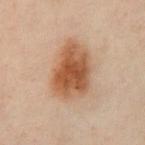Assessment: Recorded during total-body skin imaging; not selected for excision or biopsy.